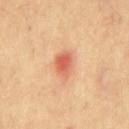Assessment: The lesion was tiled from a total-body skin photograph and was not biopsied. Image and clinical context: The lesion's longest dimension is about 3 mm. Automated image analysis of the tile measured a footprint of about 6 mm², a shape eccentricity near 0.7, and a symmetry-axis asymmetry near 0.25. It also reported a mean CIELAB color near L≈59 a*≈30 b*≈35 and a normalized border contrast of about 7.5. And it measured a within-lesion color-variation index near 4/10 and radial color variation of about 1. It also reported a nevus-likeness score of about 10/100. Imaged with cross-polarized lighting. Located on the chest. A male patient aged 68–72. A 15 mm crop from a total-body photograph taken for skin-cancer surveillance.